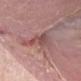From the right forearm. This is a white-light tile. A male patient about 65 years old. A 15 mm close-up extracted from a 3D total-body photography capture.